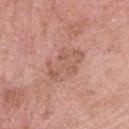Located on the head or neck. The tile uses white-light illumination. An algorithmic analysis of the crop reported a lesion area of about 13 mm², an outline eccentricity of about 0.75 (0 = round, 1 = elongated), and a shape-asymmetry score of about 0.3 (0 = symmetric). The analysis additionally found an average lesion color of about L≈58 a*≈22 b*≈28 (CIELAB), a lesion–skin lightness drop of about 7, and a normalized lesion–skin contrast near 5. The software also gave border irregularity of about 3.5 on a 0–10 scale, a color-variation rating of about 3.5/10, and radial color variation of about 1. The analysis additionally found a nevus-likeness score of about 0/100 and lesion-presence confidence of about 100/100. A male patient approximately 50 years of age. Longest diameter approximately 5 mm. A roughly 15 mm field-of-view crop from a total-body skin photograph.